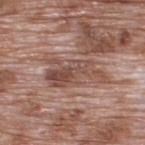Assessment:
The lesion was photographed on a routine skin check and not biopsied; there is no pathology result.
Image and clinical context:
The lesion is located on the upper back. Measured at roughly 6.5 mm in maximum diameter. A male patient aged 68–72. Cropped from a whole-body photographic skin survey; the tile spans about 15 mm.